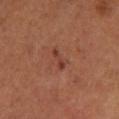  biopsy_status: not biopsied; imaged during a skin examination
  site: leg
  image:
    source: total-body photography crop
    field_of_view_mm: 15
  lesion_size:
    long_diameter_mm_approx: 2.5
  patient:
    sex: male
    age_approx: 55
  lighting: cross-polarized
  automated_metrics:
    area_mm2_approx: 2.5
    shape_asymmetry: 0.35
    nevus_likeness_0_100: 0
    lesion_detection_confidence_0_100: 100